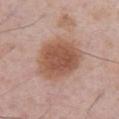Findings:
* illumination: white-light illumination
* site: the abdomen
* lesion diameter: ~6.5 mm (longest diameter)
* image: 15 mm crop, total-body photography
* patient: male, aged approximately 75
* automated metrics: a classifier nevus-likeness of about 95/100 and a lesion-detection confidence of about 100/100